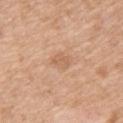Q: Was a biopsy performed?
A: no biopsy performed (imaged during a skin exam)
Q: What kind of image is this?
A: total-body-photography crop, ~15 mm field of view
Q: What is the lesion's diameter?
A: ~2.5 mm (longest diameter)
Q: Where on the body is the lesion?
A: the back
Q: Patient demographics?
A: female, aged approximately 40
Q: How was the tile lit?
A: white-light